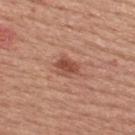Assessment:
Imaged during a routine full-body skin examination; the lesion was not biopsied and no histopathology is available.
Context:
Approximately 2.5 mm at its widest. The tile uses white-light illumination. On the back. A female patient roughly 65 years of age. A close-up tile cropped from a whole-body skin photograph, about 15 mm across. An algorithmic analysis of the crop reported an area of roughly 4.5 mm², an outline eccentricity of about 0.6 (0 = round, 1 = elongated), and a symmetry-axis asymmetry near 0.3. And it measured a border-irregularity rating of about 3/10, a color-variation rating of about 4/10, and peripheral color asymmetry of about 1.5.